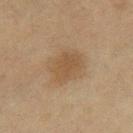No biopsy was performed on this lesion — it was imaged during a full skin examination and was not determined to be concerning. Captured under cross-polarized illumination. On the right thigh. A female subject, aged 53 to 57. The recorded lesion diameter is about 4 mm. This image is a 15 mm lesion crop taken from a total-body photograph.